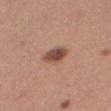The lesion was photographed on a routine skin check and not biopsied; there is no pathology result.
A lesion tile, about 15 mm wide, cut from a 3D total-body photograph.
The subject is a female in their mid- to late 30s.
Captured under white-light illumination.
About 3.5 mm across.
The lesion is located on the right thigh.